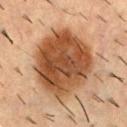This is a cross-polarized tile. A roughly 15 mm field-of-view crop from a total-body skin photograph. Automated tile analysis of the lesion measured an area of roughly 44 mm², an eccentricity of roughly 0.5, and a symmetry-axis asymmetry near 0.1. And it measured radial color variation of about 2. The analysis additionally found a nevus-likeness score of about 95/100 and lesion-presence confidence of about 100/100. The lesion is on the upper back. A male subject, in their mid- to late 50s.